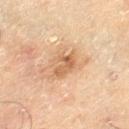No biopsy was performed on this lesion — it was imaged during a full skin examination and was not determined to be concerning. The lesion-visualizer software estimated a footprint of about 6 mm² and a symmetry-axis asymmetry near 0.3. The software also gave a lesion color around L≈50 a*≈17 b*≈31 in CIELAB and a normalized border contrast of about 6.5. And it measured a classifier nevus-likeness of about 0/100 and a detector confidence of about 100 out of 100 that the crop contains a lesion. The lesion is located on the left thigh. A roughly 15 mm field-of-view crop from a total-body skin photograph. A male subject, approximately 70 years of age. Captured under cross-polarized illumination. Longest diameter approximately 3 mm.